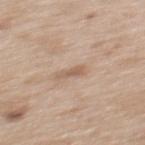{
  "biopsy_status": "not biopsied; imaged during a skin examination",
  "image": {
    "source": "total-body photography crop",
    "field_of_view_mm": 15
  },
  "lighting": "white-light",
  "site": "mid back",
  "patient": {
    "sex": "female",
    "age_approx": 40
  },
  "lesion_size": {
    "long_diameter_mm_approx": 2.5
  }
}